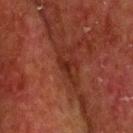Q: Is there a histopathology result?
A: no biopsy performed (imaged during a skin exam)
Q: Automated lesion metrics?
A: a shape eccentricity near 0.85 and two-axis asymmetry of about 0.5; internal color variation of about 0 on a 0–10 scale and a peripheral color-asymmetry measure near 0
Q: What are the patient's age and sex?
A: male, aged 68–72
Q: Where on the body is the lesion?
A: the head or neck
Q: What is the imaging modality?
A: ~15 mm tile from a whole-body skin photo
Q: Illumination type?
A: cross-polarized illumination
Q: Lesion size?
A: about 2.5 mm